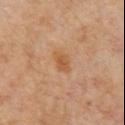Clinical impression:
Captured during whole-body skin photography for melanoma surveillance; the lesion was not biopsied.
Image and clinical context:
The recorded lesion diameter is about 3 mm. Imaged with cross-polarized lighting. Cropped from a total-body skin-imaging series; the visible field is about 15 mm. The patient is a male aged 53–57. The lesion is located on the chest. Automated image analysis of the tile measured a lesion color around L≈54 a*≈22 b*≈37 in CIELAB, a lesion–skin lightness drop of about 8, and a normalized border contrast of about 6.5.